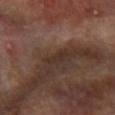Imaged with cross-polarized lighting. A male subject, aged 63–67. Cropped from a whole-body photographic skin survey; the tile spans about 15 mm. The lesion's longest dimension is about 2.5 mm. From the right forearm.